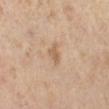No biopsy was performed on this lesion — it was imaged during a full skin examination and was not determined to be concerning.
The lesion's longest dimension is about 2.5 mm.
Located on the leg.
A female subject, aged around 40.
This is a cross-polarized tile.
A lesion tile, about 15 mm wide, cut from a 3D total-body photograph.
The lesion-visualizer software estimated an area of roughly 3.5 mm², a shape eccentricity near 0.75, and a symmetry-axis asymmetry near 0.45.